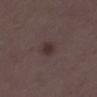lighting: white-light
site: left thigh
patient:
  sex: female
  age_approx: 35
image:
  source: total-body photography crop
  field_of_view_mm: 15